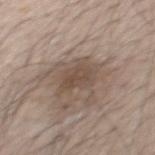Impression: No biopsy was performed on this lesion — it was imaged during a full skin examination and was not determined to be concerning. Acquisition and patient details: A 15 mm close-up extracted from a 3D total-body photography capture. The lesion-visualizer software estimated an average lesion color of about L≈48 a*≈14 b*≈24 (CIELAB) and a lesion–skin lightness drop of about 9. The software also gave a border-irregularity index near 4/10, a within-lesion color-variation index near 2.5/10, and radial color variation of about 1. It also reported an automated nevus-likeness rating near 0 out of 100 and a detector confidence of about 95 out of 100 that the crop contains a lesion. Longest diameter approximately 4 mm. Located on the back. A male patient, approximately 40 years of age.